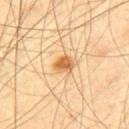Clinical impression:
No biopsy was performed on this lesion — it was imaged during a full skin examination and was not determined to be concerning.
Background:
The lesion is on the mid back. This is a cross-polarized tile. The lesion's longest dimension is about 2.5 mm. A roughly 15 mm field-of-view crop from a total-body skin photograph. An algorithmic analysis of the crop reported a footprint of about 4 mm², an outline eccentricity of about 0.45 (0 = round, 1 = elongated), and two-axis asymmetry of about 0.3. It also reported a lesion color around L≈65 a*≈23 b*≈46 in CIELAB, roughly 14 lightness units darker than nearby skin, and a lesion-to-skin contrast of about 9 (normalized; higher = more distinct). And it measured border irregularity of about 2.5 on a 0–10 scale, internal color variation of about 3.5 on a 0–10 scale, and a peripheral color-asymmetry measure near 1. The software also gave a classifier nevus-likeness of about 95/100 and a lesion-detection confidence of about 100/100. A male patient in their mid- to late 60s.